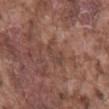Q: Was a biopsy performed?
A: catalogued during a skin exam; not biopsied
Q: Automated lesion metrics?
A: a mean CIELAB color near L≈43 a*≈19 b*≈24 and a lesion–skin lightness drop of about 7; a border-irregularity index near 5.5/10, internal color variation of about 1 on a 0–10 scale, and radial color variation of about 0.5; a nevus-likeness score of about 0/100 and lesion-presence confidence of about 75/100
Q: Illumination type?
A: white-light
Q: What is the imaging modality?
A: total-body-photography crop, ~15 mm field of view
Q: What is the anatomic site?
A: the mid back
Q: Patient demographics?
A: male, aged 73 to 77